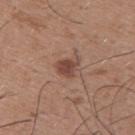| field | value |
|---|---|
| biopsy status | total-body-photography surveillance lesion; no biopsy |